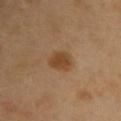Q: Was a biopsy performed?
A: total-body-photography surveillance lesion; no biopsy
Q: How was the tile lit?
A: cross-polarized
Q: What is the anatomic site?
A: the back
Q: What kind of image is this?
A: total-body-photography crop, ~15 mm field of view
Q: Who is the patient?
A: female, aged around 50